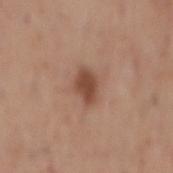workup: catalogued during a skin exam; not biopsied | illumination: white-light illumination | TBP lesion metrics: a lesion area of about 6 mm², an eccentricity of roughly 0.75, and a shape-asymmetry score of about 0.2 (0 = symmetric); a peripheral color-asymmetry measure near 0.5; a classifier nevus-likeness of about 95/100 | imaging modality: ~15 mm crop, total-body skin-cancer survey | location: the mid back | patient: male, approximately 70 years of age | size: ~3.5 mm (longest diameter).